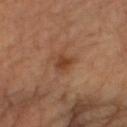Case summary:
• follow-up — no biopsy performed (imaged during a skin exam)
• subject — aged approximately 65
• lesion diameter — ~2.5 mm (longest diameter)
• location — the right upper arm
• tile lighting — cross-polarized
• image source — total-body-photography crop, ~15 mm field of view